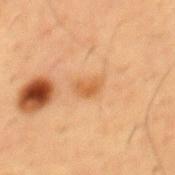Clinical impression: Part of a total-body skin-imaging series; this lesion was reviewed on a skin check and was not flagged for biopsy. Clinical summary: The recorded lesion diameter is about 2.5 mm. A male subject, about 55 years old. This is a cross-polarized tile. On the chest. A lesion tile, about 15 mm wide, cut from a 3D total-body photograph.